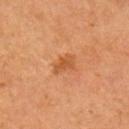Imaged during a routine full-body skin examination; the lesion was not biopsied and no histopathology is available.
A male subject, aged approximately 55.
The lesion is located on the right upper arm.
A region of skin cropped from a whole-body photographic capture, roughly 15 mm wide.